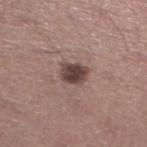biopsy status: no biopsy performed (imaged during a skin exam)
subject: male, in their mid-40s
illumination: white-light illumination
site: the left lower leg
diameter: ≈3 mm
automated lesion analysis: a shape eccentricity near 0.6; a lesion color around L≈42 a*≈17 b*≈19 in CIELAB, about 14 CIELAB-L* units darker than the surrounding skin, and a normalized border contrast of about 11; a border-irregularity index near 2/10, a color-variation rating of about 3.5/10, and a peripheral color-asymmetry measure near 1; a nevus-likeness score of about 80/100 and a detector confidence of about 100 out of 100 that the crop contains a lesion
imaging modality: ~15 mm crop, total-body skin-cancer survey